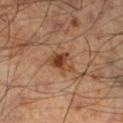| field | value |
|---|---|
| biopsy status | catalogued during a skin exam; not biopsied |
| illumination | cross-polarized |
| subject | male, roughly 55 years of age |
| location | the left lower leg |
| imaging modality | 15 mm crop, total-body photography |
| size | ≈3 mm |